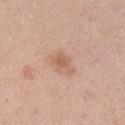Part of a total-body skin-imaging series; this lesion was reviewed on a skin check and was not flagged for biopsy.
A lesion tile, about 15 mm wide, cut from a 3D total-body photograph.
Imaged with white-light lighting.
The lesion's longest dimension is about 3.5 mm.
A male subject, aged 43 to 47.
On the right upper arm.